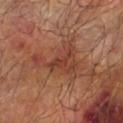biopsy status: no biopsy performed (imaged during a skin exam) | image source: total-body-photography crop, ~15 mm field of view | anatomic site: the left forearm | patient: male, aged around 60 | automated metrics: a lesion area of about 14 mm², an eccentricity of roughly 0.75, and a shape-asymmetry score of about 0.65 (0 = symmetric); a lesion color around L≈40 a*≈25 b*≈29 in CIELAB, a lesion–skin lightness drop of about 8, and a normalized border contrast of about 6.5; border irregularity of about 8 on a 0–10 scale, a color-variation rating of about 3.5/10, and peripheral color asymmetry of about 1.